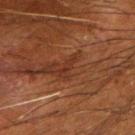biopsy status=total-body-photography surveillance lesion; no biopsy | image source=~15 mm crop, total-body skin-cancer survey | size=≈2.5 mm | lighting=cross-polarized | body site=the right forearm | automated metrics=a border-irregularity index near 4.5/10, internal color variation of about 0 on a 0–10 scale, and radial color variation of about 0; lesion-presence confidence of about 85/100 | patient=male, in their mid- to late 60s.